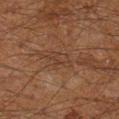Clinical impression:
No biopsy was performed on this lesion — it was imaged during a full skin examination and was not determined to be concerning.
Clinical summary:
The tile uses cross-polarized illumination. Located on the right lower leg. Automated image analysis of the tile measured a border-irregularity index near 4/10, a color-variation rating of about 2/10, and a peripheral color-asymmetry measure near 0.5. It also reported an automated nevus-likeness rating near 0 out of 100. The recorded lesion diameter is about 3.5 mm. Cropped from a whole-body photographic skin survey; the tile spans about 15 mm. A male subject, in their 60s.